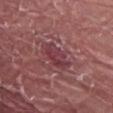This lesion was catalogued during total-body skin photography and was not selected for biopsy.
A roughly 15 mm field-of-view crop from a total-body skin photograph.
Imaged with white-light lighting.
Automated image analysis of the tile measured an eccentricity of roughly 0.85 and a shape-asymmetry score of about 0.3 (0 = symmetric). The analysis additionally found a border-irregularity rating of about 3.5/10, a color-variation rating of about 3.5/10, and peripheral color asymmetry of about 1. The analysis additionally found a lesion-detection confidence of about 95/100.
Approximately 4 mm at its widest.
The subject is a male aged 38 to 42.
From the abdomen.